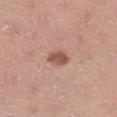<tbp_lesion>
<biopsy_status>not biopsied; imaged during a skin examination</biopsy_status>
<site>right lower leg</site>
<lesion_size>
  <long_diameter_mm_approx>3.0</long_diameter_mm_approx>
</lesion_size>
<patient>
  <sex>female</sex>
  <age_approx>35</age_approx>
</patient>
<lighting>white-light</lighting>
<image>
  <source>total-body photography crop</source>
  <field_of_view_mm>15</field_of_view_mm>
</image>
</tbp_lesion>